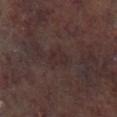Assessment: No biopsy was performed on this lesion — it was imaged during a full skin examination and was not determined to be concerning. Clinical summary: A 15 mm close-up extracted from a 3D total-body photography capture. Captured under cross-polarized illumination. The lesion is located on the leg. A male subject aged 63–67.